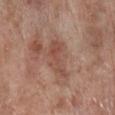Captured during whole-body skin photography for melanoma surveillance; the lesion was not biopsied.
A male subject, aged approximately 70.
The lesion is on the right lower leg.
Cropped from a whole-body photographic skin survey; the tile spans about 15 mm.
The tile uses white-light illumination.